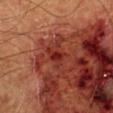Imaged during a routine full-body skin examination; the lesion was not biopsied and no histopathology is available. The lesion is on the left lower leg. A male subject about 60 years old. A 15 mm close-up extracted from a 3D total-body photography capture.